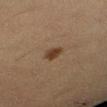workup = no biopsy performed (imaged during a skin exam) | patient = female, aged approximately 30 | anatomic site = the left lower leg | acquisition = total-body-photography crop, ~15 mm field of view.